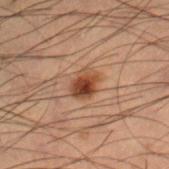No biopsy was performed on this lesion — it was imaged during a full skin examination and was not determined to be concerning.
On the right lower leg.
This is a cross-polarized tile.
A male patient aged 53–57.
The recorded lesion diameter is about 3 mm.
A lesion tile, about 15 mm wide, cut from a 3D total-body photograph.